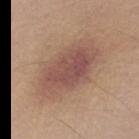Longest diameter approximately 8 mm. A male patient in their mid-60s. Located on the upper back. A 15 mm crop from a total-body photograph taken for skin-cancer surveillance. The tile uses white-light illumination. Automated image analysis of the tile measured a mean CIELAB color near L≈51 a*≈20 b*≈25 and a normalized border contrast of about 7.5. The software also gave border irregularity of about 2.5 on a 0–10 scale, a color-variation rating of about 4.5/10, and radial color variation of about 1.